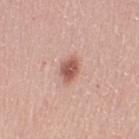Part of a total-body skin-imaging series; this lesion was reviewed on a skin check and was not flagged for biopsy.
Approximately 3 mm at its widest.
A female patient about 55 years old.
Cropped from a whole-body photographic skin survey; the tile spans about 15 mm.
The lesion is located on the right upper arm.
Imaged with white-light lighting.